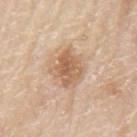Q: Was a biopsy performed?
A: imaged on a skin check; not biopsied
Q: Where on the body is the lesion?
A: the left upper arm
Q: Patient demographics?
A: male, aged approximately 80
Q: What lighting was used for the tile?
A: white-light
Q: How large is the lesion?
A: about 4 mm
Q: What is the imaging modality?
A: total-body-photography crop, ~15 mm field of view